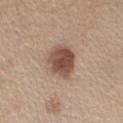Longest diameter approximately 4 mm. This image is a 15 mm lesion crop taken from a total-body photograph. A male patient aged 53 to 57. The lesion is on the right upper arm. Imaged with white-light lighting.